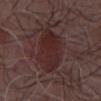biopsy_status: not biopsied; imaged during a skin examination
automated_metrics:
  area_mm2_approx: 19.0
  eccentricity: 0.85
  cielab_L: 26
  cielab_a: 19
  cielab_b: 18
  vs_skin_contrast_norm: 7.5
  border_irregularity_0_10: 2.5
  color_variation_0_10: 3.0
  peripheral_color_asymmetry: 1.0
  nevus_likeness_0_100: 40
  lesion_detection_confidence_0_100: 100
lesion_size:
  long_diameter_mm_approx: 7.0
patient:
  sex: male
  age_approx: 55
lighting: white-light
image:
  source: total-body photography crop
  field_of_view_mm: 15
site: front of the torso